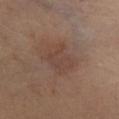follow-up — catalogued during a skin exam; not biopsied | location — the right leg | image source — 15 mm crop, total-body photography | patient — female, roughly 65 years of age | lesion size — ~4 mm (longest diameter) | illumination — cross-polarized.